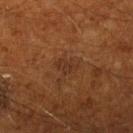{
  "biopsy_status": "not biopsied; imaged during a skin examination",
  "lesion_size": {
    "long_diameter_mm_approx": 2.5
  },
  "patient": {
    "sex": "male",
    "age_approx": 60
  },
  "site": "right lower leg",
  "image": {
    "source": "total-body photography crop",
    "field_of_view_mm": 15
  }
}